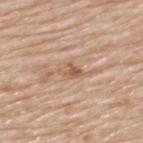| feature | finding |
|---|---|
| biopsy status | no biopsy performed (imaged during a skin exam) |
| body site | the upper back |
| patient | male, roughly 75 years of age |
| image source | ~15 mm crop, total-body skin-cancer survey |
| illumination | white-light illumination |
| diameter | ~3 mm (longest diameter) |